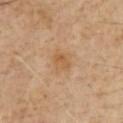  biopsy_status: not biopsied; imaged during a skin examination
  automated_metrics:
    cielab_L: 55
    cielab_a: 18
    cielab_b: 36
    vs_skin_darker_L: 6.0
  patient:
    sex: male
    age_approx: 70
  image:
    source: total-body photography crop
    field_of_view_mm: 15
  site: chest
  lesion_size:
    long_diameter_mm_approx: 3.0